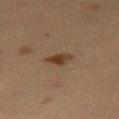follow-up = catalogued during a skin exam; not biopsied
anatomic site = the right thigh
subject = female, about 40 years old
acquisition = 15 mm crop, total-body photography